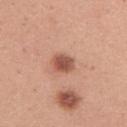Q: Was a biopsy performed?
A: no biopsy performed (imaged during a skin exam)
Q: How large is the lesion?
A: about 2.5 mm
Q: What is the imaging modality?
A: 15 mm crop, total-body photography
Q: What are the patient's age and sex?
A: female, aged 28 to 32
Q: What is the anatomic site?
A: the right upper arm